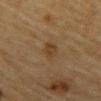Background: The tile uses cross-polarized illumination. From the abdomen. The recorded lesion diameter is about 3 mm. A 15 mm close-up tile from a total-body photography series done for melanoma screening. The subject is a male in their mid-80s or older.